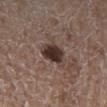Clinical impression:
This lesion was catalogued during total-body skin photography and was not selected for biopsy.
Background:
The patient is a female aged around 70. A 15 mm crop from a total-body photograph taken for skin-cancer surveillance. Imaged with white-light lighting. The lesion-visualizer software estimated an area of roughly 7.5 mm², a shape eccentricity near 0.7, and a symmetry-axis asymmetry near 0.15. And it measured a mean CIELAB color near L≈32 a*≈16 b*≈19 and a normalized border contrast of about 13.5. And it measured an automated nevus-likeness rating near 85 out of 100 and a lesion-detection confidence of about 100/100. From the right lower leg. The lesion's longest dimension is about 3.5 mm.